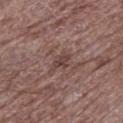Impression:
Imaged during a routine full-body skin examination; the lesion was not biopsied and no histopathology is available.